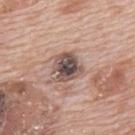lesion diameter: ≈3.5 mm | TBP lesion metrics: a footprint of about 9 mm² and an eccentricity of roughly 0.35; border irregularity of about 2.5 on a 0–10 scale, a within-lesion color-variation index near 7.5/10, and a peripheral color-asymmetry measure near 2; an automated nevus-likeness rating near 100 out of 100 and lesion-presence confidence of about 100/100 | anatomic site: the back | patient: male, aged around 70 | imaging modality: 15 mm crop, total-body photography.